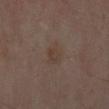Q: Was this lesion biopsied?
A: imaged on a skin check; not biopsied
Q: What is the anatomic site?
A: the left forearm
Q: What lighting was used for the tile?
A: cross-polarized
Q: What is the lesion's diameter?
A: about 3 mm
Q: What is the imaging modality?
A: ~15 mm crop, total-body skin-cancer survey
Q: Who is the patient?
A: male, in their 50s